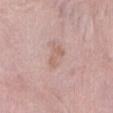Q: Was this lesion biopsied?
A: catalogued during a skin exam; not biopsied